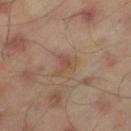The lesion was tiled from a total-body skin photograph and was not biopsied.
Captured under cross-polarized illumination.
The subject is a male roughly 45 years of age.
A lesion tile, about 15 mm wide, cut from a 3D total-body photograph.
An algorithmic analysis of the crop reported an area of roughly 5.5 mm² and a shape eccentricity near 0.65.
Longest diameter approximately 3 mm.
On the left thigh.